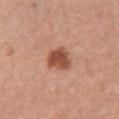  biopsy_status: not biopsied; imaged during a skin examination
  patient:
    sex: female
    age_approx: 60
  lighting: white-light
  site: right upper arm
  lesion_size:
    long_diameter_mm_approx: 3.5
  image:
    source: total-body photography crop
    field_of_view_mm: 15